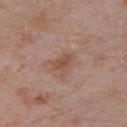Q: Is there a histopathology result?
A: catalogued during a skin exam; not biopsied
Q: Who is the patient?
A: male, in their mid- to late 50s
Q: What is the imaging modality?
A: 15 mm crop, total-body photography
Q: What is the anatomic site?
A: the right upper arm